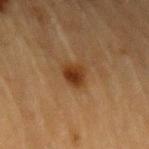Clinical summary:
Automated image analysis of the tile measured a footprint of about 6 mm², a shape eccentricity near 0.4, and a symmetry-axis asymmetry near 0.3. The software also gave an average lesion color of about L≈32 a*≈19 b*≈32 (CIELAB), about 10 CIELAB-L* units darker than the surrounding skin, and a lesion-to-skin contrast of about 9.5 (normalized; higher = more distinct). It also reported an automated nevus-likeness rating near 95 out of 100. The subject is a male in their mid- to late 80s or older. A 15 mm close-up extracted from a 3D total-body photography capture. From the left upper arm. Approximately 3 mm at its widest.